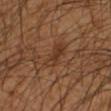<tbp_lesion>
  <biopsy_status>not biopsied; imaged during a skin examination</biopsy_status>
  <patient>
    <sex>male</sex>
    <age_approx>50</age_approx>
  </patient>
  <image>
    <source>total-body photography crop</source>
    <field_of_view_mm>15</field_of_view_mm>
  </image>
  <site>left forearm</site>
</tbp_lesion>